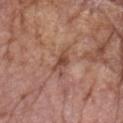Captured during whole-body skin photography for melanoma surveillance; the lesion was not biopsied. From the left upper arm. This is a white-light tile. The recorded lesion diameter is about 3 mm. A region of skin cropped from a whole-body photographic capture, roughly 15 mm wide. The patient is a male aged 83 or over. The total-body-photography lesion software estimated a classifier nevus-likeness of about 0/100 and a lesion-detection confidence of about 100/100.